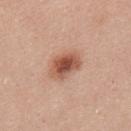<tbp_lesion>
<biopsy_status>not biopsied; imaged during a skin examination</biopsy_status>
<patient>
  <sex>male</sex>
  <age_approx>25</age_approx>
</patient>
<lighting>white-light</lighting>
<lesion_size>
  <long_diameter_mm_approx>4.5</long_diameter_mm_approx>
</lesion_size>
<site>upper back</site>
<image>
  <source>total-body photography crop</source>
  <field_of_view_mm>15</field_of_view_mm>
</image>
</tbp_lesion>